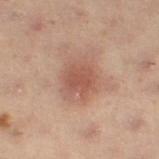Recorded during total-body skin imaging; not selected for excision or biopsy.
The subject is a female aged around 55.
On the left leg.
Imaged with cross-polarized lighting.
Cropped from a total-body skin-imaging series; the visible field is about 15 mm.
About 4 mm across.
Automated tile analysis of the lesion measured a lesion area of about 11 mm² and two-axis asymmetry of about 0.25.